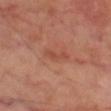Recorded during total-body skin imaging; not selected for excision or biopsy.
A male subject aged 68–72.
A 15 mm crop from a total-body photograph taken for skin-cancer surveillance.
Captured under cross-polarized illumination.
From the left thigh.